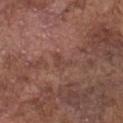This lesion was catalogued during total-body skin photography and was not selected for biopsy. From the chest. The subject is a male aged around 75. A 15 mm close-up extracted from a 3D total-body photography capture.